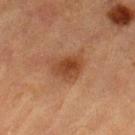Case summary:
• notes — imaged on a skin check; not biopsied
• patient — male, roughly 85 years of age or older
• location — the left thigh
• diameter — ≈4 mm
• automated lesion analysis — an area of roughly 9.5 mm², an eccentricity of roughly 0.7, and two-axis asymmetry of about 0.2
• lighting — cross-polarized illumination
• image — ~15 mm tile from a whole-body skin photo